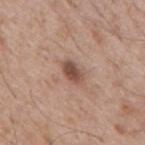Assessment:
The lesion was photographed on a routine skin check and not biopsied; there is no pathology result.
Context:
Approximately 3 mm at its widest. The subject is a male aged 43 to 47. Automated image analysis of the tile measured about 13 CIELAB-L* units darker than the surrounding skin and a normalized border contrast of about 9. The software also gave border irregularity of about 2 on a 0–10 scale, a color-variation rating of about 4.5/10, and radial color variation of about 1.5. On the mid back. A region of skin cropped from a whole-body photographic capture, roughly 15 mm wide.